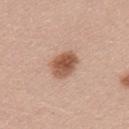This image is a 15 mm lesion crop taken from a total-body photograph. The subject is a male aged 28–32.